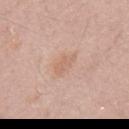Part of a total-body skin-imaging series; this lesion was reviewed on a skin check and was not flagged for biopsy. The lesion is on the mid back. The subject is a male roughly 55 years of age. A region of skin cropped from a whole-body photographic capture, roughly 15 mm wide.